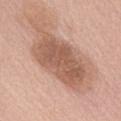Case summary:
* biopsy status: catalogued during a skin exam; not biopsied
* image source: ~15 mm tile from a whole-body skin photo
* subject: female, aged 58 to 62
* lighting: white-light illumination
* size: ~7.5 mm (longest diameter)
* image-analysis metrics: a mean CIELAB color near L≈57 a*≈21 b*≈29 and a lesion–skin lightness drop of about 12
* body site: the mid back